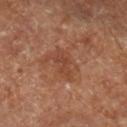Q: Was a biopsy performed?
A: total-body-photography surveillance lesion; no biopsy
Q: Patient demographics?
A: aged approximately 65
Q: How was this image acquired?
A: total-body-photography crop, ~15 mm field of view
Q: Illumination type?
A: cross-polarized
Q: How large is the lesion?
A: ~3.5 mm (longest diameter)
Q: What did automated image analysis measure?
A: a lesion color around L≈42 a*≈23 b*≈30 in CIELAB, roughly 7 lightness units darker than nearby skin, and a normalized border contrast of about 5.5; a border-irregularity rating of about 4.5/10, a within-lesion color-variation index near 2/10, and peripheral color asymmetry of about 0.5
Q: Lesion location?
A: the right lower leg